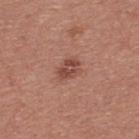Captured during whole-body skin photography for melanoma surveillance; the lesion was not biopsied.